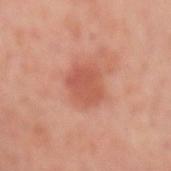Assessment:
This lesion was catalogued during total-body skin photography and was not selected for biopsy.
Background:
The patient is a male about 50 years old. Automated tile analysis of the lesion measured border irregularity of about 2.5 on a 0–10 scale, a color-variation rating of about 3/10, and radial color variation of about 1. A roughly 15 mm field-of-view crop from a total-body skin photograph. The lesion is on the left forearm. Imaged with cross-polarized lighting.